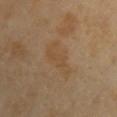| feature | finding |
|---|---|
| biopsy status | imaged on a skin check; not biopsied |
| body site | the front of the torso |
| patient | female, roughly 50 years of age |
| lesion size | ~5 mm (longest diameter) |
| imaging modality | total-body-photography crop, ~15 mm field of view |
| automated metrics | a shape eccentricity near 0.85; a lesion–skin lightness drop of about 5 and a normalized lesion–skin contrast near 4.5; an automated nevus-likeness rating near 0 out of 100 and a detector confidence of about 100 out of 100 that the crop contains a lesion |